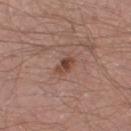Clinical impression: Imaged during a routine full-body skin examination; the lesion was not biopsied and no histopathology is available. Context: On the right thigh. Longest diameter approximately 2.5 mm. A roughly 15 mm field-of-view crop from a total-body skin photograph. The tile uses white-light illumination. The patient is a male roughly 65 years of age.